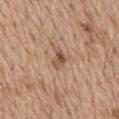follow-up = imaged on a skin check; not biopsied
image = ~15 mm tile from a whole-body skin photo
patient = male, in their 70s
site = the mid back
illumination = white-light
lesion diameter = ~2.5 mm (longest diameter)